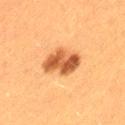follow-up=no biopsy performed (imaged during a skin exam) | acquisition=15 mm crop, total-body photography | patient=female, aged around 40 | body site=the left thigh | illumination=cross-polarized | diameter=about 4.5 mm | automated lesion analysis=a lesion area of about 11 mm², a shape eccentricity near 0.65, and two-axis asymmetry of about 0.2; a mean CIELAB color near L≈48 a*≈24 b*≈37; a border-irregularity rating of about 2/10, internal color variation of about 6.5 on a 0–10 scale, and radial color variation of about 2; an automated nevus-likeness rating near 95 out of 100 and a lesion-detection confidence of about 100/100.